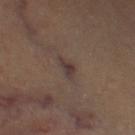{
  "biopsy_status": "not biopsied; imaged during a skin examination",
  "site": "left thigh",
  "lesion_size": {
    "long_diameter_mm_approx": 2.5
  },
  "patient": {
    "age_approx": 60
  },
  "image": {
    "source": "total-body photography crop",
    "field_of_view_mm": 15
  }
}